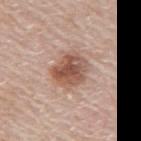biopsy status: imaged on a skin check; not biopsied | tile lighting: white-light | acquisition: ~15 mm crop, total-body skin-cancer survey | anatomic site: the arm | lesion diameter: ≈4 mm | subject: female, aged 48 to 52.